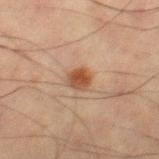Impression: The lesion was tiled from a total-body skin photograph and was not biopsied. Background: A close-up tile cropped from a whole-body skin photograph, about 15 mm across. From the leg. A male subject, in their mid-60s. The tile uses cross-polarized illumination.